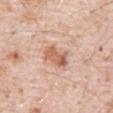biopsy_status: not biopsied; imaged during a skin examination
patient:
  sex: male
  age_approx: 80
image:
  source: total-body photography crop
  field_of_view_mm: 15
automated_metrics:
  area_mm2_approx: 7.5
  eccentricity: 0.85
  shape_asymmetry: 0.3
  cielab_L: 62
  cielab_a: 22
  cielab_b: 31
  vs_skin_darker_L: 11.0
  vs_skin_contrast_norm: 7.5
lesion_size:
  long_diameter_mm_approx: 4.0
lighting: white-light
site: abdomen